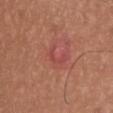Clinical impression:
Imaged during a routine full-body skin examination; the lesion was not biopsied and no histopathology is available.
Context:
A male subject aged 23 to 27. From the chest. This image is a 15 mm lesion crop taken from a total-body photograph. This is a white-light tile. The lesion's longest dimension is about 3.5 mm. Automated tile analysis of the lesion measured a lesion area of about 4 mm², an outline eccentricity of about 0.9 (0 = round, 1 = elongated), and a shape-asymmetry score of about 0.65 (0 = symmetric). The software also gave a border-irregularity index near 8/10 and radial color variation of about 0. The software also gave an automated nevus-likeness rating near 0 out of 100 and a lesion-detection confidence of about 100/100.